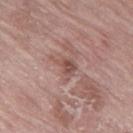Case summary:
* workup — imaged on a skin check; not biopsied
* subject — female, aged around 75
* illumination — white-light illumination
* anatomic site — the left thigh
* imaging modality — total-body-photography crop, ~15 mm field of view
* lesion diameter — ~3.5 mm (longest diameter)